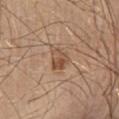This lesion was catalogued during total-body skin photography and was not selected for biopsy. On the abdomen. A male patient, aged 38–42. Approximately 3 mm at its widest. A lesion tile, about 15 mm wide, cut from a 3D total-body photograph.